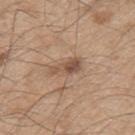* notes: total-body-photography surveillance lesion; no biopsy
* patient: male, aged approximately 50
* imaging modality: ~15 mm tile from a whole-body skin photo
* anatomic site: the upper back